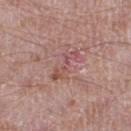Impression:
The lesion was tiled from a total-body skin photograph and was not biopsied.
Acquisition and patient details:
The lesion's longest dimension is about 4.5 mm. A 15 mm close-up extracted from a 3D total-body photography capture. On the left lower leg. Automated image analysis of the tile measured a mean CIELAB color near L≈52 a*≈24 b*≈22 and a lesion–skin lightness drop of about 7. The software also gave an automated nevus-likeness rating near 0 out of 100 and a lesion-detection confidence of about 100/100. The subject is a male in their mid- to late 60s. Imaged with white-light lighting.